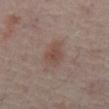The patient is in their mid- to late 50s.
The lesion is located on the abdomen.
A roughly 15 mm field-of-view crop from a total-body skin photograph.